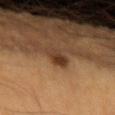Recorded during total-body skin imaging; not selected for excision or biopsy.
The patient is a male roughly 60 years of age.
The lesion is located on the arm.
The lesion-visualizer software estimated a footprint of about 4.5 mm², an outline eccentricity of about 0.85 (0 = round, 1 = elongated), and a symmetry-axis asymmetry near 0.2. It also reported a lesion-to-skin contrast of about 9 (normalized; higher = more distinct). It also reported a color-variation rating of about 5.5/10 and radial color variation of about 2. It also reported a classifier nevus-likeness of about 95/100.
The lesion's longest dimension is about 3.5 mm.
The tile uses cross-polarized illumination.
A close-up tile cropped from a whole-body skin photograph, about 15 mm across.